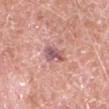| key | value |
|---|---|
| follow-up | no biopsy performed (imaged during a skin exam) |
| diameter | about 3 mm |
| automated metrics | an automated nevus-likeness rating near 0 out of 100 |
| anatomic site | the chest |
| lighting | white-light illumination |
| image source | ~15 mm tile from a whole-body skin photo |
| subject | male, aged approximately 65 |